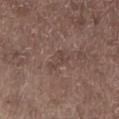{"biopsy_status": "not biopsied; imaged during a skin examination", "site": "left lower leg", "patient": {"sex": "male", "age_approx": 70}, "lesion_size": {"long_diameter_mm_approx": 4.0}, "image": {"source": "total-body photography crop", "field_of_view_mm": 15}, "lighting": "white-light", "automated_metrics": {"cielab_L": 43, "cielab_a": 16, "cielab_b": 21, "vs_skin_darker_L": 5.0, "nevus_likeness_0_100": 0, "lesion_detection_confidence_0_100": 60}}